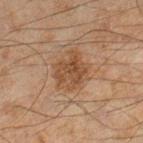This lesion was catalogued during total-body skin photography and was not selected for biopsy.
Located on the leg.
Imaged with cross-polarized lighting.
An algorithmic analysis of the crop reported a footprint of about 13 mm². The analysis additionally found a mean CIELAB color near L≈39 a*≈16 b*≈27, roughly 7 lightness units darker than nearby skin, and a lesion-to-skin contrast of about 7 (normalized; higher = more distinct). The analysis additionally found internal color variation of about 3.5 on a 0–10 scale and radial color variation of about 1.
A male subject aged 43 to 47.
A lesion tile, about 15 mm wide, cut from a 3D total-body photograph.